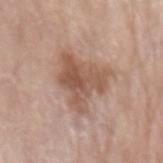workup — no biopsy performed (imaged during a skin exam) | lighting — white-light | body site — the arm | image — ~15 mm crop, total-body skin-cancer survey | subject — male, aged around 80 | size — ≈5 mm.